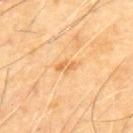biopsy status = catalogued during a skin exam; not biopsied
acquisition = total-body-photography crop, ~15 mm field of view
subject = male, in their 70s
location = the upper back